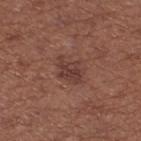On the leg.
The recorded lesion diameter is about 3.5 mm.
A female patient, aged around 55.
This image is a 15 mm lesion crop taken from a total-body photograph.